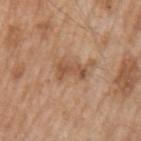biopsy status: catalogued during a skin exam; not biopsied
subject: male, roughly 65 years of age
body site: the left upper arm
acquisition: ~15 mm tile from a whole-body skin photo